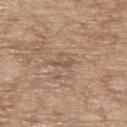The lesion was tiled from a total-body skin photograph and was not biopsied. A male subject aged 63 to 67. The lesion is on the upper back. The tile uses white-light illumination. A close-up tile cropped from a whole-body skin photograph, about 15 mm across. The recorded lesion diameter is about 3 mm. The lesion-visualizer software estimated a nevus-likeness score of about 0/100.